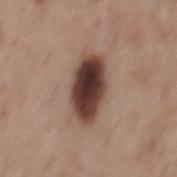Findings:
- notes: imaged on a skin check; not biopsied
- imaging modality: 15 mm crop, total-body photography
- tile lighting: white-light
- lesion size: ~6 mm (longest diameter)
- body site: the mid back
- patient: male, roughly 55 years of age